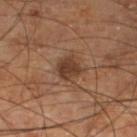{
  "biopsy_status": "not biopsied; imaged during a skin examination",
  "site": "left lower leg",
  "patient": {
    "sex": "male",
    "age_approx": 70
  },
  "lighting": "cross-polarized",
  "image": {
    "source": "total-body photography crop",
    "field_of_view_mm": 15
  }
}